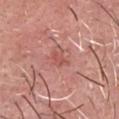Assessment:
Part of a total-body skin-imaging series; this lesion was reviewed on a skin check and was not flagged for biopsy.
Image and clinical context:
A 15 mm crop from a total-body photograph taken for skin-cancer surveillance. Automated tile analysis of the lesion measured a footprint of about 3 mm², an eccentricity of roughly 0.9, and two-axis asymmetry of about 0.4. The software also gave a within-lesion color-variation index near 1/10 and peripheral color asymmetry of about 0. The recorded lesion diameter is about 3 mm. On the head or neck. A male patient aged 48 to 52. The tile uses white-light illumination.